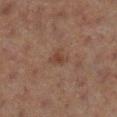{
  "lighting": "cross-polarized",
  "automated_metrics": {
    "area_mm2_approx": 4.0,
    "eccentricity": 0.55,
    "shape_asymmetry": 0.35,
    "cielab_L": 34,
    "cielab_a": 16,
    "cielab_b": 23,
    "border_irregularity_0_10": 3.5,
    "color_variation_0_10": 2.0,
    "peripheral_color_asymmetry": 0.5,
    "nevus_likeness_0_100": 5,
    "lesion_detection_confidence_0_100": 100
  },
  "patient": {
    "sex": "female",
    "age_approx": 40
  },
  "image": {
    "source": "total-body photography crop",
    "field_of_view_mm": 15
  },
  "lesion_size": {
    "long_diameter_mm_approx": 2.5
  },
  "site": "right lower leg"
}